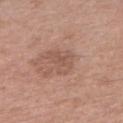Part of a total-body skin-imaging series; this lesion was reviewed on a skin check and was not flagged for biopsy. On the arm. The tile uses white-light illumination. The total-body-photography lesion software estimated a footprint of about 6 mm², an eccentricity of roughly 0.8, and a symmetry-axis asymmetry near 0.5. The software also gave a lesion color around L≈53 a*≈20 b*≈27 in CIELAB, roughly 7 lightness units darker than nearby skin, and a lesion-to-skin contrast of about 5 (normalized; higher = more distinct). And it measured a border-irregularity rating of about 6/10, internal color variation of about 2 on a 0–10 scale, and a peripheral color-asymmetry measure near 0.5. The analysis additionally found a nevus-likeness score of about 0/100 and lesion-presence confidence of about 100/100. A female subject in their mid- to late 40s. Longest diameter approximately 4 mm. A 15 mm crop from a total-body photograph taken for skin-cancer surveillance.